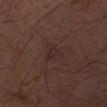| feature | finding |
|---|---|
| notes | imaged on a skin check; not biopsied |
| image-analysis metrics | an area of roughly 4.5 mm²; a lesion color around L≈27 a*≈17 b*≈18 in CIELAB, about 4 CIELAB-L* units darker than the surrounding skin, and a lesion-to-skin contrast of about 5 (normalized; higher = more distinct); a border-irregularity index near 3.5/10; a classifier nevus-likeness of about 25/100 |
| illumination | cross-polarized |
| site | the left forearm |
| acquisition | ~15 mm tile from a whole-body skin photo |
| lesion diameter | about 3 mm |